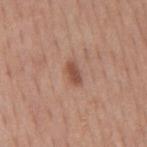This lesion was catalogued during total-body skin photography and was not selected for biopsy.
A 15 mm close-up tile from a total-body photography series done for melanoma screening.
Captured under white-light illumination.
The recorded lesion diameter is about 2.5 mm.
The patient is a male aged approximately 60.
The lesion is on the mid back.